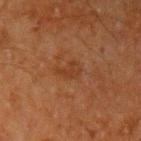Findings:
* follow-up — catalogued during a skin exam; not biopsied
* image — total-body-photography crop, ~15 mm field of view
* illumination — cross-polarized
* diameter — ~3 mm (longest diameter)
* patient — male, aged approximately 60
* anatomic site — the left upper arm
* automated metrics — a lesion area of about 3.5 mm², a shape eccentricity near 0.85, and a symmetry-axis asymmetry near 0.4; border irregularity of about 4.5 on a 0–10 scale, a within-lesion color-variation index near 1/10, and a peripheral color-asymmetry measure near 0.5; an automated nevus-likeness rating near 0 out of 100